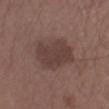biopsy status: total-body-photography surveillance lesion; no biopsy
acquisition: ~15 mm crop, total-body skin-cancer survey
tile lighting: white-light
lesion size: ≈5.5 mm
TBP lesion metrics: an area of roughly 18 mm² and an outline eccentricity of about 0.55 (0 = round, 1 = elongated); a border-irregularity rating of about 2.5/10; an automated nevus-likeness rating near 10 out of 100 and a detector confidence of about 100 out of 100 that the crop contains a lesion
site: the right lower leg
patient: male, aged 73–77